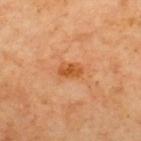workup: no biopsy performed (imaged during a skin exam); lighting: cross-polarized illumination; patient: aged approximately 65; image source: 15 mm crop, total-body photography; location: the upper back.